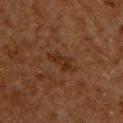Assessment: The lesion was tiled from a total-body skin photograph and was not biopsied. Context: Located on the front of the torso. Captured under cross-polarized illumination. A male patient, aged 58–62. A 15 mm crop from a total-body photograph taken for skin-cancer surveillance.